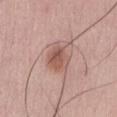Clinical impression: Captured during whole-body skin photography for melanoma surveillance; the lesion was not biopsied. Clinical summary: The recorded lesion diameter is about 3.5 mm. Imaged with white-light lighting. A 15 mm close-up tile from a total-body photography series done for melanoma screening. The subject is a female aged approximately 45. The lesion is on the abdomen.